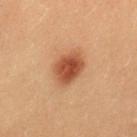No biopsy was performed on this lesion — it was imaged during a full skin examination and was not determined to be concerning.
The recorded lesion diameter is about 4 mm.
The tile uses cross-polarized illumination.
From the mid back.
A female patient in their 20s.
Cropped from a total-body skin-imaging series; the visible field is about 15 mm.